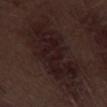notes = no biopsy performed (imaged during a skin exam)
subject = male, roughly 70 years of age
image = ~15 mm tile from a whole-body skin photo
anatomic site = the left thigh
tile lighting = white-light
image-analysis metrics = a lesion color around L≈18 a*≈14 b*≈14 in CIELAB, roughly 6 lightness units darker than nearby skin, and a normalized border contrast of about 9; a within-lesion color-variation index near 3.5/10 and a peripheral color-asymmetry measure near 1; a nevus-likeness score of about 0/100 and a detector confidence of about 55 out of 100 that the crop contains a lesion
lesion size = ≈14 mm